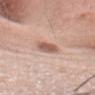Assessment:
This lesion was catalogued during total-body skin photography and was not selected for biopsy.
Acquisition and patient details:
The subject is a male in their mid- to late 30s. A close-up tile cropped from a whole-body skin photograph, about 15 mm across. This is a white-light tile. The lesion is located on the head or neck. Approximately 3 mm at its widest.